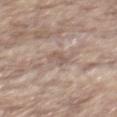size: about 2.5 mm; body site: the mid back; lighting: white-light; imaging modality: ~15 mm crop, total-body skin-cancer survey; subject: male, aged approximately 65.